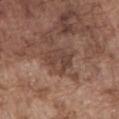<tbp_lesion>
  <biopsy_status>not biopsied; imaged during a skin examination</biopsy_status>
  <image>
    <source>total-body photography crop</source>
    <field_of_view_mm>15</field_of_view_mm>
  </image>
  <lighting>white-light</lighting>
  <site>abdomen</site>
  <patient>
    <sex>male</sex>
    <age_approx>75</age_approx>
  </patient>
</tbp_lesion>